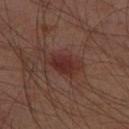The lesion is located on the right thigh. A male patient about 60 years old. Cropped from a whole-body photographic skin survey; the tile spans about 15 mm.